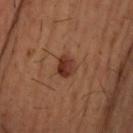Impression:
Captured during whole-body skin photography for melanoma surveillance; the lesion was not biopsied.
Background:
This is a cross-polarized tile. Cropped from a total-body skin-imaging series; the visible field is about 15 mm. The lesion is located on the upper back. A male subject, aged approximately 35. Measured at roughly 2.5 mm in maximum diameter.